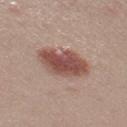Notes:
- follow-up: total-body-photography surveillance lesion; no biopsy
- illumination: white-light illumination
- patient: female, aged 23–27
- automated metrics: a symmetry-axis asymmetry near 0.2; a border-irregularity rating of about 2.5/10, a within-lesion color-variation index near 5/10, and radial color variation of about 1.5; an automated nevus-likeness rating near 100 out of 100
- anatomic site: the right thigh
- acquisition: total-body-photography crop, ~15 mm field of view